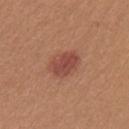Impression:
Imaged during a routine full-body skin examination; the lesion was not biopsied and no histopathology is available.
Clinical summary:
About 4 mm across. The lesion is located on the left upper arm. A close-up tile cropped from a whole-body skin photograph, about 15 mm across. Imaged with white-light lighting. A female patient about 25 years old.